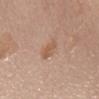Q: Is there a histopathology result?
A: no biopsy performed (imaged during a skin exam)
Q: Lesion size?
A: ~2.5 mm (longest diameter)
Q: Lesion location?
A: the abdomen
Q: Illumination type?
A: white-light
Q: What did automated image analysis measure?
A: a footprint of about 4 mm², an outline eccentricity of about 0.75 (0 = round, 1 = elongated), and a shape-asymmetry score of about 0.25 (0 = symmetric); a mean CIELAB color near L≈56 a*≈19 b*≈31, roughly 8 lightness units darker than nearby skin, and a lesion-to-skin contrast of about 6 (normalized; higher = more distinct)
Q: Who is the patient?
A: female, aged 58–62
Q: What is the imaging modality?
A: ~15 mm crop, total-body skin-cancer survey